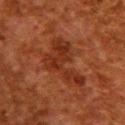This lesion was catalogued during total-body skin photography and was not selected for biopsy.
The recorded lesion diameter is about 7 mm.
Cropped from a total-body skin-imaging series; the visible field is about 15 mm.
The patient is a female in their 50s.
Imaged with cross-polarized lighting.
The lesion is on the back.